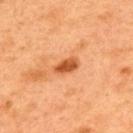Findings:
- workup · catalogued during a skin exam; not biopsied
- location · the back
- image source · 15 mm crop, total-body photography
- patient · male, aged 48 to 52